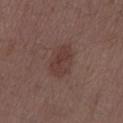{"biopsy_status": "not biopsied; imaged during a skin examination"}